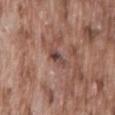Q: Was this lesion biopsied?
A: no biopsy performed (imaged during a skin exam)
Q: Lesion size?
A: ≈3 mm
Q: What kind of image is this?
A: 15 mm crop, total-body photography
Q: Illumination type?
A: white-light
Q: Lesion location?
A: the lower back
Q: What are the patient's age and sex?
A: male, aged approximately 75